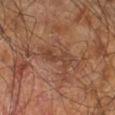Clinical impression: The lesion was tiled from a total-body skin photograph and was not biopsied. Clinical summary: Cropped from a whole-body photographic skin survey; the tile spans about 15 mm. On the right leg. Captured under cross-polarized illumination. Measured at roughly 5 mm in maximum diameter. A male subject, in their 60s.